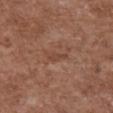Imaged with white-light lighting.
The recorded lesion diameter is about 3 mm.
The subject is a female aged 73–77.
The lesion-visualizer software estimated a lesion area of about 3 mm², an outline eccentricity of about 0.85 (0 = round, 1 = elongated), and two-axis asymmetry of about 0.4. It also reported a mean CIELAB color near L≈44 a*≈21 b*≈28. It also reported a border-irregularity rating of about 4/10 and a within-lesion color-variation index near 0.5/10. The analysis additionally found a classifier nevus-likeness of about 0/100.
A 15 mm crop from a total-body photograph taken for skin-cancer surveillance.
The lesion is on the chest.